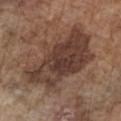Q: Was a biopsy performed?
A: catalogued during a skin exam; not biopsied
Q: How was this image acquired?
A: 15 mm crop, total-body photography
Q: Where on the body is the lesion?
A: the chest
Q: What lighting was used for the tile?
A: white-light illumination
Q: Who is the patient?
A: male, aged around 75
Q: How large is the lesion?
A: ~9.5 mm (longest diameter)